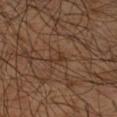  biopsy_status: not biopsied; imaged during a skin examination
  lighting: cross-polarized
  automated_metrics:
    area_mm2_approx: 3.0
    shape_asymmetry: 0.5
    cielab_L: 35
    cielab_a: 18
    cielab_b: 28
    vs_skin_darker_L: 6.0
    vs_skin_contrast_norm: 6.0
    color_variation_0_10: 0.0
    peripheral_color_asymmetry: 0.0
  image:
    source: total-body photography crop
    field_of_view_mm: 15
  site: right forearm
  patient:
    sex: male
    age_approx: 50